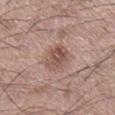{"biopsy_status": "not biopsied; imaged during a skin examination", "image": {"source": "total-body photography crop", "field_of_view_mm": 15}, "patient": {"sex": "male", "age_approx": 60}, "site": "right lower leg"}